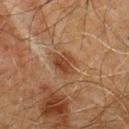Assessment: Captured during whole-body skin photography for melanoma surveillance; the lesion was not biopsied. Acquisition and patient details: Automated tile analysis of the lesion measured an average lesion color of about L≈40 a*≈21 b*≈32 (CIELAB) and a normalized border contrast of about 7.5. And it measured border irregularity of about 4 on a 0–10 scale, a within-lesion color-variation index near 3.5/10, and peripheral color asymmetry of about 1. It also reported a classifier nevus-likeness of about 70/100 and lesion-presence confidence of about 100/100. This is a cross-polarized tile. A lesion tile, about 15 mm wide, cut from a 3D total-body photograph. A male subject, in their 60s. The lesion is located on the chest. About 3 mm across.